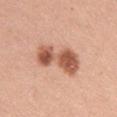Q: Is there a histopathology result?
A: total-body-photography surveillance lesion; no biopsy
Q: Lesion location?
A: the left upper arm
Q: How large is the lesion?
A: ~6 mm (longest diameter)
Q: Patient demographics?
A: female, in their mid-30s
Q: What kind of image is this?
A: 15 mm crop, total-body photography